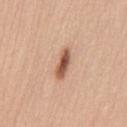notes: imaged on a skin check; not biopsied | anatomic site: the mid back | subject: male, roughly 60 years of age | image: total-body-photography crop, ~15 mm field of view | size: about 4 mm.